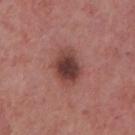Image and clinical context:
Imaged with white-light lighting. The subject is a female about 40 years old. A region of skin cropped from a whole-body photographic capture, roughly 15 mm wide. Located on the right lower leg. The lesion's longest dimension is about 4.5 mm.